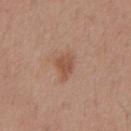Q: Is there a histopathology result?
A: imaged on a skin check; not biopsied
Q: Automated lesion metrics?
A: a nevus-likeness score of about 55/100
Q: How was the tile lit?
A: white-light
Q: Patient demographics?
A: male, aged 28–32
Q: Lesion size?
A: about 3 mm
Q: What is the anatomic site?
A: the chest
Q: How was this image acquired?
A: ~15 mm tile from a whole-body skin photo